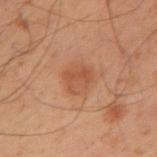This lesion was catalogued during total-body skin photography and was not selected for biopsy. The patient is a male roughly 50 years of age. Cropped from a whole-body photographic skin survey; the tile spans about 15 mm. From the left arm. Imaged with cross-polarized lighting. Automated image analysis of the tile measured an area of roughly 8.5 mm². The analysis additionally found a lesion color around L≈43 a*≈20 b*≈29 in CIELAB, roughly 6 lightness units darker than nearby skin, and a lesion-to-skin contrast of about 5 (normalized; higher = more distinct). The analysis additionally found an automated nevus-likeness rating near 20 out of 100 and a lesion-detection confidence of about 100/100. Approximately 3.5 mm at its widest.